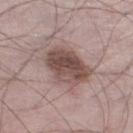<case>
  <biopsy_status>not biopsied; imaged during a skin examination</biopsy_status>
  <patient>
    <sex>male</sex>
    <age_approx>70</age_approx>
  </patient>
  <image>
    <source>total-body photography crop</source>
    <field_of_view_mm>15</field_of_view_mm>
  </image>
  <lighting>white-light</lighting>
  <site>left lower leg</site>
  <automated_metrics>
    <cielab_L>49</cielab_L>
    <cielab_a>17</cielab_a>
    <cielab_b>21</cielab_b>
    <vs_skin_darker_L>13.0</vs_skin_darker_L>
    <vs_skin_contrast_norm>9.5</vs_skin_contrast_norm>
    <nevus_likeness_0_100>40</nevus_likeness_0_100>
    <lesion_detection_confidence_0_100>100</lesion_detection_confidence_0_100>
  </automated_metrics>
</case>